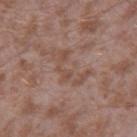Part of a total-body skin-imaging series; this lesion was reviewed on a skin check and was not flagged for biopsy. The lesion is located on the right thigh. A close-up tile cropped from a whole-body skin photograph, about 15 mm across. Approximately 5 mm at its widest. A male subject, about 45 years old. This is a white-light tile.